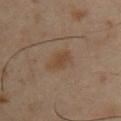{
  "biopsy_status": "not biopsied; imaged during a skin examination",
  "site": "chest",
  "patient": {
    "sex": "male",
    "age_approx": 40
  },
  "image": {
    "source": "total-body photography crop",
    "field_of_view_mm": 15
  },
  "lesion_size": {
    "long_diameter_mm_approx": 3.5
  },
  "automated_metrics": {
    "cielab_L": 43,
    "cielab_a": 15,
    "cielab_b": 29,
    "vs_skin_contrast_norm": 6.0,
    "border_irregularity_0_10": 2.5,
    "color_variation_0_10": 2.5,
    "peripheral_color_asymmetry": 1.0,
    "lesion_detection_confidence_0_100": 100
  },
  "lighting": "cross-polarized"
}